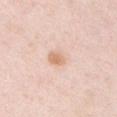The recorded lesion diameter is about 3.5 mm. Located on the front of the torso. The lesion-visualizer software estimated a lesion area of about 7.5 mm² and an eccentricity of roughly 0.55. A male patient about 35 years old. A roughly 15 mm field-of-view crop from a total-body skin photograph.